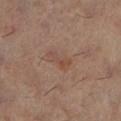Recorded during total-body skin imaging; not selected for excision or biopsy.
Automated image analysis of the tile measured an eccentricity of roughly 0.9 and a symmetry-axis asymmetry near 0.7. And it measured an average lesion color of about L≈48 a*≈20 b*≈27 (CIELAB), roughly 6 lightness units darker than nearby skin, and a lesion-to-skin contrast of about 5 (normalized; higher = more distinct). The software also gave border irregularity of about 7.5 on a 0–10 scale, a color-variation rating of about 0/10, and peripheral color asymmetry of about 0.
A female subject aged around 60.
Cropped from a total-body skin-imaging series; the visible field is about 15 mm.
The tile uses cross-polarized illumination.
The lesion's longest dimension is about 3.5 mm.
Located on the left lower leg.